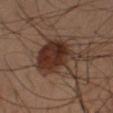biopsy_status: not biopsied; imaged during a skin examination
image:
  source: total-body photography crop
  field_of_view_mm: 15
lighting: cross-polarized
site: left arm
patient:
  sex: male
  age_approx: 50
lesion_size:
  long_diameter_mm_approx: 8.0